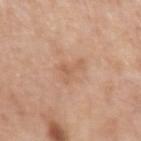Q: Was a biopsy performed?
A: total-body-photography surveillance lesion; no biopsy
Q: Where on the body is the lesion?
A: the left upper arm
Q: Who is the patient?
A: male, aged around 55
Q: How was this image acquired?
A: 15 mm crop, total-body photography
Q: Illumination type?
A: white-light illumination
Q: Lesion size?
A: ~3.5 mm (longest diameter)
Q: What did automated image analysis measure?
A: a mean CIELAB color near L≈59 a*≈21 b*≈33 and a lesion-to-skin contrast of about 5 (normalized; higher = more distinct); a border-irregularity rating of about 7/10, internal color variation of about 2 on a 0–10 scale, and a peripheral color-asymmetry measure near 1; a nevus-likeness score of about 0/100 and a detector confidence of about 100 out of 100 that the crop contains a lesion